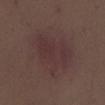Q: Was this lesion biopsied?
A: catalogued during a skin exam; not biopsied
Q: What is the lesion's diameter?
A: ~7.5 mm (longest diameter)
Q: What is the imaging modality?
A: total-body-photography crop, ~15 mm field of view
Q: What is the anatomic site?
A: the mid back
Q: What are the patient's age and sex?
A: male, about 30 years old
Q: What did automated image analysis measure?
A: a footprint of about 23 mm², an eccentricity of roughly 0.8, and two-axis asymmetry of about 0.3; an average lesion color of about L≈33 a*≈17 b*≈16 (CIELAB), roughly 5 lightness units darker than nearby skin, and a normalized border contrast of about 5; border irregularity of about 3.5 on a 0–10 scale, a color-variation rating of about 2.5/10, and radial color variation of about 0.5; an automated nevus-likeness rating near 10 out of 100